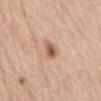Notes:
* workup · catalogued during a skin exam; not biopsied
* subject · female, aged 63–67
* diameter · ≈2.5 mm
* site · the abdomen
* image · total-body-photography crop, ~15 mm field of view
* TBP lesion metrics · an area of roughly 4 mm², an eccentricity of roughly 0.75, and a shape-asymmetry score of about 0.25 (0 = symmetric); lesion-presence confidence of about 100/100